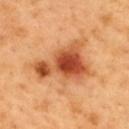  biopsy_status: not biopsied; imaged during a skin examination
  lighting: cross-polarized
  patient:
    sex: male
    age_approx: 50
  site: mid back
  image:
    source: total-body photography crop
    field_of_view_mm: 15
  lesion_size:
    long_diameter_mm_approx: 8.0
  automated_metrics:
    cielab_L: 55
    cielab_a: 29
    cielab_b: 41
    vs_skin_darker_L: 15.0
    vs_skin_contrast_norm: 9.5
    border_irregularity_0_10: 6.0
    color_variation_0_10: 10.0
    peripheral_color_asymmetry: 3.5